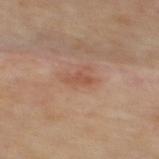Imaged during a routine full-body skin examination; the lesion was not biopsied and no histopathology is available. This is a cross-polarized tile. The lesion-visualizer software estimated a border-irregularity rating of about 4/10, a color-variation rating of about 0/10, and peripheral color asymmetry of about 0. And it measured a nevus-likeness score of about 5/100 and a detector confidence of about 100 out of 100 that the crop contains a lesion. Longest diameter approximately 2.5 mm. The lesion is on the mid back. Cropped from a total-body skin-imaging series; the visible field is about 15 mm.